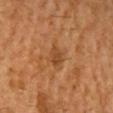Clinical impression:
No biopsy was performed on this lesion — it was imaged during a full skin examination and was not determined to be concerning.
Context:
A male subject aged 68 to 72. A close-up tile cropped from a whole-body skin photograph, about 15 mm across. The lesion is located on the head or neck.